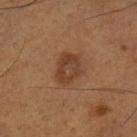biopsy status = no biopsy performed (imaged during a skin exam) | lighting = cross-polarized illumination | size = about 4 mm | patient = male, aged 53 to 57 | image = 15 mm crop, total-body photography | location = the left lower leg.